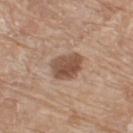Imaged during a routine full-body skin examination; the lesion was not biopsied and no histopathology is available. A close-up tile cropped from a whole-body skin photograph, about 15 mm across. A female subject, aged 73 to 77. The lesion is on the right thigh.